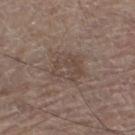{"biopsy_status": "not biopsied; imaged during a skin examination", "patient": {"sex": "male", "age_approx": 65}, "automated_metrics": {"cielab_L": 45, "cielab_a": 14, "cielab_b": 22, "vs_skin_darker_L": 6.0}, "lighting": "white-light", "site": "left lower leg", "image": {"source": "total-body photography crop", "field_of_view_mm": 15}}